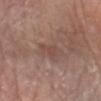Impression: Imaged during a routine full-body skin examination; the lesion was not biopsied and no histopathology is available. Acquisition and patient details: Automated tile analysis of the lesion measured an area of roughly 3.5 mm², an outline eccentricity of about 0.85 (0 = round, 1 = elongated), and a shape-asymmetry score of about 0.5 (0 = symmetric). The software also gave a mean CIELAB color near L≈47 a*≈19 b*≈23, a lesion–skin lightness drop of about 6, and a lesion-to-skin contrast of about 5 (normalized; higher = more distinct). It also reported border irregularity of about 5 on a 0–10 scale and radial color variation of about 0. A lesion tile, about 15 mm wide, cut from a 3D total-body photograph. A male subject, aged 78 to 82. The lesion is on the left forearm. The recorded lesion diameter is about 3 mm. The tile uses white-light illumination.